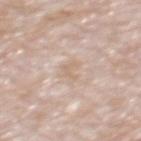workup: catalogued during a skin exam; not biopsied.